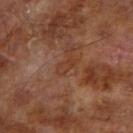| key | value |
|---|---|
| notes | imaged on a skin check; not biopsied |
| tile lighting | cross-polarized |
| diameter | ≈2.5 mm |
| body site | the right upper arm |
| patient | male, aged 63–67 |
| TBP lesion metrics | an eccentricity of roughly 0.85 and a shape-asymmetry score of about 0.3 (0 = symmetric); a mean CIELAB color near L≈37 a*≈22 b*≈29, about 5 CIELAB-L* units darker than the surrounding skin, and a normalized border contrast of about 5; a border-irregularity rating of about 3/10, a within-lesion color-variation index near 0.5/10, and peripheral color asymmetry of about 0 |
| imaging modality | 15 mm crop, total-body photography |